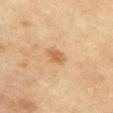| field | value |
|---|---|
| biopsy status | total-body-photography surveillance lesion; no biopsy |
| lighting | cross-polarized illumination |
| acquisition | ~15 mm tile from a whole-body skin photo |
| lesion diameter | about 3 mm |
| site | the chest |
| subject | female, aged approximately 50 |